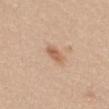Part of a total-body skin-imaging series; this lesion was reviewed on a skin check and was not flagged for biopsy. A female patient aged 23–27. Automated image analysis of the tile measured roughly 10 lightness units darker than nearby skin and a normalized lesion–skin contrast near 7. On the back. A lesion tile, about 15 mm wide, cut from a 3D total-body photograph. Longest diameter approximately 2.5 mm. Imaged with white-light lighting.